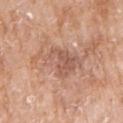Imaged during a routine full-body skin examination; the lesion was not biopsied and no histopathology is available.
Automated image analysis of the tile measured a footprint of about 14 mm², an outline eccentricity of about 0.9 (0 = round, 1 = elongated), and two-axis asymmetry of about 0.35. The analysis additionally found border irregularity of about 5.5 on a 0–10 scale, internal color variation of about 4 on a 0–10 scale, and peripheral color asymmetry of about 1.5. It also reported a classifier nevus-likeness of about 0/100 and lesion-presence confidence of about 100/100.
A 15 mm crop from a total-body photograph taken for skin-cancer surveillance.
Captured under white-light illumination.
Approximately 7 mm at its widest.
The lesion is located on the arm.
The patient is a female aged 73 to 77.